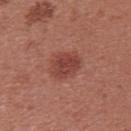Impression:
This lesion was catalogued during total-body skin photography and was not selected for biopsy.
Image and clinical context:
Imaged with white-light lighting. The lesion's longest dimension is about 3.5 mm. From the upper back. Automated tile analysis of the lesion measured an area of roughly 8.5 mm², an outline eccentricity of about 0.65 (0 = round, 1 = elongated), and a shape-asymmetry score of about 0.2 (0 = symmetric). The software also gave an average lesion color of about L≈43 a*≈27 b*≈26 (CIELAB), about 9 CIELAB-L* units darker than the surrounding skin, and a normalized lesion–skin contrast near 7.5. The software also gave a border-irregularity rating of about 2/10, a within-lesion color-variation index near 3/10, and radial color variation of about 1. A lesion tile, about 15 mm wide, cut from a 3D total-body photograph. A female subject, aged around 25.